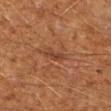Clinical impression: Recorded during total-body skin imaging; not selected for excision or biopsy. Image and clinical context: The lesion is on the right upper arm. Approximately 3 mm at its widest. Imaged with cross-polarized lighting. The subject is a male aged 58 to 62. A region of skin cropped from a whole-body photographic capture, roughly 15 mm wide.